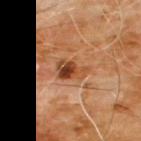Imaged during a routine full-body skin examination; the lesion was not biopsied and no histopathology is available. The lesion's longest dimension is about 3.5 mm. A male subject approximately 60 years of age. This image is a 15 mm lesion crop taken from a total-body photograph. The total-body-photography lesion software estimated a lesion color around L≈44 a*≈25 b*≈36 in CIELAB, about 13 CIELAB-L* units darker than the surrounding skin, and a lesion-to-skin contrast of about 9.5 (normalized; higher = more distinct). The lesion is located on the front of the torso.